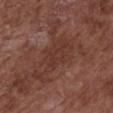Captured during whole-body skin photography for melanoma surveillance; the lesion was not biopsied. The lesion is on the chest. This is a white-light tile. The lesion-visualizer software estimated a footprint of about 4.5 mm², a shape eccentricity near 0.9, and a shape-asymmetry score of about 0.45 (0 = symmetric). The analysis additionally found an average lesion color of about L≈34 a*≈23 b*≈24 (CIELAB) and a lesion-to-skin contrast of about 4.5 (normalized; higher = more distinct). The software also gave border irregularity of about 5.5 on a 0–10 scale and peripheral color asymmetry of about 0.5. Longest diameter approximately 3.5 mm. A roughly 15 mm field-of-view crop from a total-body skin photograph. A female patient about 80 years old.